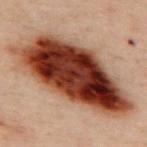Q: Was a biopsy performed?
A: catalogued during a skin exam; not biopsied
Q: What kind of image is this?
A: ~15 mm tile from a whole-body skin photo
Q: What is the lesion's diameter?
A: about 14.5 mm
Q: Patient demographics?
A: male, aged 48 to 52
Q: What did automated image analysis measure?
A: about 21 CIELAB-L* units darker than the surrounding skin and a normalized border contrast of about 17
Q: Illumination type?
A: cross-polarized illumination
Q: Lesion location?
A: the upper back